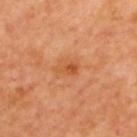Impression: The lesion was photographed on a routine skin check and not biopsied; there is no pathology result. Context: A roughly 15 mm field-of-view crop from a total-body skin photograph. A male subject aged 63 to 67. On the upper back.